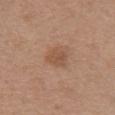Captured during whole-body skin photography for melanoma surveillance; the lesion was not biopsied.
The patient is a female in their 50s.
A 15 mm close-up tile from a total-body photography series done for melanoma screening.
The lesion is on the chest.
This is a white-light tile.
Automated image analysis of the tile measured an area of roughly 5 mm² and an eccentricity of roughly 0.8. The analysis additionally found an automated nevus-likeness rating near 35 out of 100 and a detector confidence of about 100 out of 100 that the crop contains a lesion.